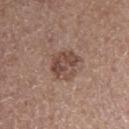– notes · total-body-photography surveillance lesion; no biopsy
– site · the left upper arm
– subject · male, roughly 60 years of age
– diameter · ~3.5 mm (longest diameter)
– lighting · white-light
– image source · ~15 mm crop, total-body skin-cancer survey
– TBP lesion metrics · a lesion area of about 9.5 mm², an outline eccentricity of about 0.45 (0 = round, 1 = elongated), and a shape-asymmetry score of about 0.2 (0 = symmetric); a lesion color around L≈46 a*≈18 b*≈25 in CIELAB, a lesion–skin lightness drop of about 10, and a normalized lesion–skin contrast near 7.5; a color-variation rating of about 5/10